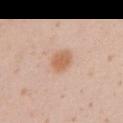<lesion>
<biopsy_status>not biopsied; imaged during a skin examination</biopsy_status>
<lesion_size>
  <long_diameter_mm_approx>3.0</long_diameter_mm_approx>
</lesion_size>
<image>
  <source>total-body photography crop</source>
  <field_of_view_mm>15</field_of_view_mm>
</image>
<patient>
  <sex>female</sex>
  <age_approx>20</age_approx>
</patient>
<lighting>white-light</lighting>
<site>arm</site>
</lesion>